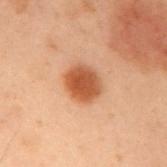Q: Is there a histopathology result?
A: no biopsy performed (imaged during a skin exam)
Q: How was this image acquired?
A: ~15 mm tile from a whole-body skin photo
Q: What is the anatomic site?
A: the right upper arm
Q: What did automated image analysis measure?
A: a lesion area of about 10 mm², an outline eccentricity of about 0.55 (0 = round, 1 = elongated), and a shape-asymmetry score of about 0.1 (0 = symmetric); a lesion color around L≈44 a*≈23 b*≈32 in CIELAB and a normalized border contrast of about 10
Q: Patient demographics?
A: male, aged approximately 55